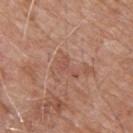Part of a total-body skin-imaging series; this lesion was reviewed on a skin check and was not flagged for biopsy. The lesion's longest dimension is about 3 mm. A 15 mm close-up extracted from a 3D total-body photography capture. A male patient, aged 78–82. The lesion is located on the back. Imaged with white-light lighting.